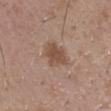Imaged during a routine full-body skin examination; the lesion was not biopsied and no histopathology is available. The patient is a male aged approximately 50. On the right forearm. Approximately 3.5 mm at its widest. Cropped from a total-body skin-imaging series; the visible field is about 15 mm. Imaged with white-light lighting.